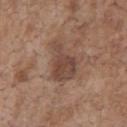This lesion was catalogued during total-body skin photography and was not selected for biopsy.
This is a white-light tile.
The subject is a male roughly 70 years of age.
A roughly 15 mm field-of-view crop from a total-body skin photograph.
The lesion's longest dimension is about 5 mm.
The lesion is on the chest.